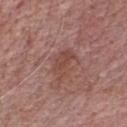No biopsy was performed on this lesion — it was imaged during a full skin examination and was not determined to be concerning. The lesion is on the chest. A male subject aged 68 to 72. This image is a 15 mm lesion crop taken from a total-body photograph. The tile uses white-light illumination. The lesion-visualizer software estimated a footprint of about 6.5 mm², an eccentricity of roughly 0.85, and a shape-asymmetry score of about 0.35 (0 = symmetric). And it measured roughly 7 lightness units darker than nearby skin and a normalized lesion–skin contrast near 5.5. The analysis additionally found a nevus-likeness score of about 0/100 and a detector confidence of about 100 out of 100 that the crop contains a lesion. Approximately 4 mm at its widest.